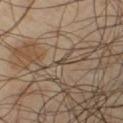Q: What is the anatomic site?
A: the leg
Q: Automated lesion metrics?
A: a lesion area of about 0.5 mm², an outline eccentricity of about 0.85 (0 = round, 1 = elongated), and a shape-asymmetry score of about 0.4 (0 = symmetric); a border-irregularity index near 3/10, internal color variation of about 0 on a 0–10 scale, and radial color variation of about 0; a classifier nevus-likeness of about 0/100 and lesion-presence confidence of about 0/100
Q: Lesion size?
A: about 1 mm
Q: How was this image acquired?
A: total-body-photography crop, ~15 mm field of view
Q: What are the patient's age and sex?
A: male, aged 38–42
Q: What lighting was used for the tile?
A: cross-polarized